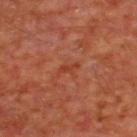  patient:
    sex: male
    age_approx: 65
  lesion_size:
    long_diameter_mm_approx: 2.5
  image:
    source: total-body photography crop
    field_of_view_mm: 15
  lighting: cross-polarized
  site: upper back